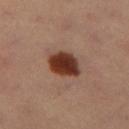biopsy status — imaged on a skin check; not biopsied
TBP lesion metrics — a footprint of about 9.5 mm² and an outline eccentricity of about 0.6 (0 = round, 1 = elongated); an average lesion color of about L≈36 a*≈23 b*≈28 (CIELAB), about 18 CIELAB-L* units darker than the surrounding skin, and a lesion-to-skin contrast of about 14.5 (normalized; higher = more distinct); a lesion-detection confidence of about 100/100
subject — female, aged 38 to 42
location — the right leg
tile lighting — cross-polarized
acquisition — ~15 mm crop, total-body skin-cancer survey
size — ~4 mm (longest diameter)